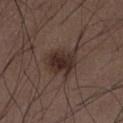The lesion was photographed on a routine skin check and not biopsied; there is no pathology result. A male subject, about 50 years old. Measured at roughly 5 mm in maximum diameter. The lesion is located on the abdomen. This image is a 15 mm lesion crop taken from a total-body photograph.